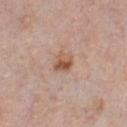subject: male, aged 48 to 52
automated lesion analysis: a footprint of about 5 mm² and a shape eccentricity near 0.5; an average lesion color of about L≈55 a*≈20 b*≈31 (CIELAB), roughly 10 lightness units darker than nearby skin, and a lesion-to-skin contrast of about 8 (normalized; higher = more distinct); border irregularity of about 2 on a 0–10 scale, internal color variation of about 7 on a 0–10 scale, and radial color variation of about 2.5; a classifier nevus-likeness of about 85/100 and a lesion-detection confidence of about 100/100
illumination: white-light illumination
body site: the chest
lesion diameter: about 2.5 mm
imaging modality: ~15 mm crop, total-body skin-cancer survey